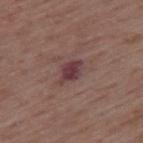{
  "patient": {
    "sex": "male",
    "age_approx": 55
  },
  "site": "back",
  "image": {
    "source": "total-body photography crop",
    "field_of_view_mm": 15
  }
}